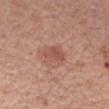* workup — total-body-photography surveillance lesion; no biopsy
* tile lighting — white-light illumination
* image source — total-body-photography crop, ~15 mm field of view
* diameter — about 3.5 mm
* anatomic site — the right upper arm
* automated metrics — a lesion area of about 4.5 mm² and a shape eccentricity near 0.8
* subject — male, aged approximately 60